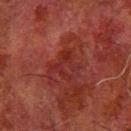Clinical impression:
No biopsy was performed on this lesion — it was imaged during a full skin examination and was not determined to be concerning.
Context:
Automated image analysis of the tile measured a border-irregularity rating of about 6/10, a within-lesion color-variation index near 4.5/10, and a peripheral color-asymmetry measure near 1.5. And it measured a nevus-likeness score of about 0/100 and a detector confidence of about 85 out of 100 that the crop contains a lesion. A lesion tile, about 15 mm wide, cut from a 3D total-body photograph. A male subject aged around 80. The lesion is located on the arm. Measured at roughly 3.5 mm in maximum diameter. This is a cross-polarized tile.